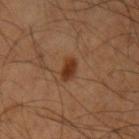No biopsy was performed on this lesion — it was imaged during a full skin examination and was not determined to be concerning. Longest diameter approximately 2.5 mm. On the right upper arm. A region of skin cropped from a whole-body photographic capture, roughly 15 mm wide. Captured under cross-polarized illumination. The patient is a male aged 58–62.